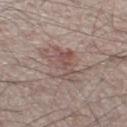Case summary:
– biopsy status — imaged on a skin check; not biopsied